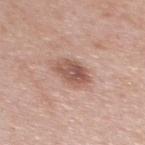follow-up = total-body-photography surveillance lesion; no biopsy | subject = female, roughly 35 years of age | image source = ~15 mm tile from a whole-body skin photo | illumination = white-light | diameter = about 3.5 mm | body site = the upper back.